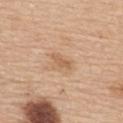Case summary:
• acquisition — total-body-photography crop, ~15 mm field of view
• subject — female, aged 63–67
• anatomic site — the upper back